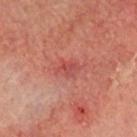Case summary:
* notes — catalogued during a skin exam; not biopsied
* illumination — cross-polarized
* image source — ~15 mm tile from a whole-body skin photo
* anatomic site — the head or neck
* subject — male, in their mid-60s
* lesion size — ≈3 mm